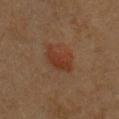Q: Was a biopsy performed?
A: no biopsy performed (imaged during a skin exam)
Q: How was this image acquired?
A: 15 mm crop, total-body photography
Q: How large is the lesion?
A: about 4.5 mm
Q: What are the patient's age and sex?
A: female, aged 38–42
Q: What did automated image analysis measure?
A: a mean CIELAB color near L≈31 a*≈19 b*≈26, a lesion–skin lightness drop of about 6, and a normalized border contrast of about 6.5; a nevus-likeness score of about 70/100 and a lesion-detection confidence of about 100/100
Q: Where on the body is the lesion?
A: the chest